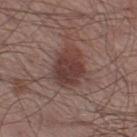Q: Was a biopsy performed?
A: imaged on a skin check; not biopsied
Q: What is the anatomic site?
A: the right lower leg
Q: What did automated image analysis measure?
A: lesion-presence confidence of about 100/100
Q: What are the patient's age and sex?
A: male, aged 53 to 57
Q: Lesion size?
A: about 4.5 mm
Q: How was this image acquired?
A: total-body-photography crop, ~15 mm field of view